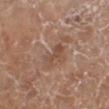This lesion was catalogued during total-body skin photography and was not selected for biopsy.
Automated image analysis of the tile measured an average lesion color of about L≈49 a*≈19 b*≈28 (CIELAB), a lesion–skin lightness drop of about 7, and a normalized border contrast of about 5.5. The software also gave a border-irregularity rating of about 5/10, a color-variation rating of about 2/10, and peripheral color asymmetry of about 0.5.
A 15 mm crop from a total-body photograph taken for skin-cancer surveillance.
A female patient, about 75 years old.
The lesion is located on the left lower leg.
This is a white-light tile.
Approximately 3 mm at its widest.